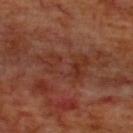Recorded during total-body skin imaging; not selected for excision or biopsy.
The lesion-visualizer software estimated a lesion color around L≈32 a*≈24 b*≈27 in CIELAB, a lesion–skin lightness drop of about 5, and a lesion-to-skin contrast of about 5.5 (normalized; higher = more distinct). The software also gave border irregularity of about 6 on a 0–10 scale, internal color variation of about 4.5 on a 0–10 scale, and a peripheral color-asymmetry measure near 1. And it measured a classifier nevus-likeness of about 0/100 and lesion-presence confidence of about 95/100.
A male patient, about 70 years old.
From the upper back.
A 15 mm close-up tile from a total-body photography series done for melanoma screening.
The tile uses cross-polarized illumination.